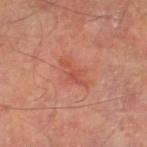Case summary:
• notes — total-body-photography surveillance lesion; no biopsy
• lighting — cross-polarized
• image source — total-body-photography crop, ~15 mm field of view
• subject — male, aged 63–67
• location — the leg
• lesion diameter — ≈4 mm